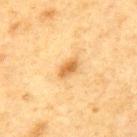The recorded lesion diameter is about 2.5 mm. Located on the mid back. A male subject, roughly 75 years of age. A 15 mm close-up tile from a total-body photography series done for melanoma screening.